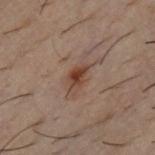| field | value |
|---|---|
| biopsy status | total-body-photography surveillance lesion; no biopsy |
| subject | male, aged around 30 |
| anatomic site | the chest |
| acquisition | ~15 mm crop, total-body skin-cancer survey |